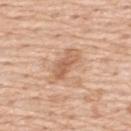Part of a total-body skin-imaging series; this lesion was reviewed on a skin check and was not flagged for biopsy. A male patient, in their 60s. The lesion is located on the upper back. A 15 mm crop from a total-body photograph taken for skin-cancer surveillance.